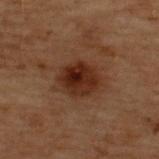workup: imaged on a skin check; not biopsied | patient: male, aged 68–72 | illumination: cross-polarized illumination | diameter: ~4 mm (longest diameter) | automated lesion analysis: a lesion color around L≈20 a*≈17 b*≈22 in CIELAB, roughly 9 lightness units darker than nearby skin, and a normalized lesion–skin contrast near 10.5; a classifier nevus-likeness of about 90/100 and a detector confidence of about 100 out of 100 that the crop contains a lesion | body site: the upper back | acquisition: ~15 mm crop, total-body skin-cancer survey.